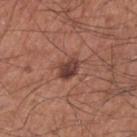The lesion was photographed on a routine skin check and not biopsied; there is no pathology result.
Cropped from a whole-body photographic skin survey; the tile spans about 15 mm.
Automated tile analysis of the lesion measured internal color variation of about 5 on a 0–10 scale and a peripheral color-asymmetry measure near 2.
On the arm.
A male patient aged 63–67.
Measured at roughly 3 mm in maximum diameter.